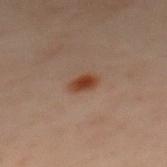| key | value |
|---|---|
| biopsy status | total-body-photography surveillance lesion; no biopsy |
| patient | female, approximately 55 years of age |
| imaging modality | ~15 mm crop, total-body skin-cancer survey |
| body site | the upper back |